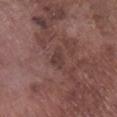<record>
  <biopsy_status>not biopsied; imaged during a skin examination</biopsy_status>
  <image>
    <source>total-body photography crop</source>
    <field_of_view_mm>15</field_of_view_mm>
  </image>
  <patient>
    <sex>male</sex>
    <age_approx>75</age_approx>
  </patient>
  <site>right lower leg</site>
</record>